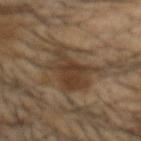No biopsy was performed on this lesion — it was imaged during a full skin examination and was not determined to be concerning. A roughly 15 mm field-of-view crop from a total-body skin photograph. The lesion is on the head or neck. The patient is a male aged 53 to 57.